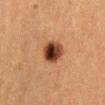Q: Is there a histopathology result?
A: total-body-photography surveillance lesion; no biopsy
Q: How large is the lesion?
A: ≈3.5 mm
Q: What are the patient's age and sex?
A: female, about 40 years old
Q: Lesion location?
A: the abdomen
Q: Illumination type?
A: cross-polarized
Q: What kind of image is this?
A: ~15 mm tile from a whole-body skin photo